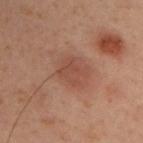A male subject aged approximately 45.
Automated tile analysis of the lesion measured a nevus-likeness score of about 65/100 and lesion-presence confidence of about 100/100.
This is a cross-polarized tile.
A 15 mm crop from a total-body photograph taken for skin-cancer surveillance.
From the upper back.
Approximately 3 mm at its widest.